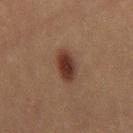Imaged with cross-polarized lighting.
A 15 mm crop from a total-body photograph taken for skin-cancer surveillance.
Longest diameter approximately 4 mm.
On the right thigh.
Automated tile analysis of the lesion measured a footprint of about 7 mm², an outline eccentricity of about 0.85 (0 = round, 1 = elongated), and a symmetry-axis asymmetry near 0.15. The software also gave a mean CIELAB color near L≈31 a*≈19 b*≈24, roughly 11 lightness units darker than nearby skin, and a normalized border contrast of about 11. It also reported a lesion-detection confidence of about 100/100.
A female patient approximately 20 years of age.